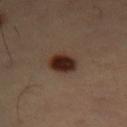Q: What is the anatomic site?
A: the left thigh
Q: Lesion size?
A: ≈3.5 mm
Q: How was this image acquired?
A: total-body-photography crop, ~15 mm field of view
Q: What lighting was used for the tile?
A: cross-polarized
Q: What did automated image analysis measure?
A: a footprint of about 7 mm², an outline eccentricity of about 0.6 (0 = round, 1 = elongated), and a symmetry-axis asymmetry near 0.15; an automated nevus-likeness rating near 100 out of 100
Q: Who is the patient?
A: male, approximately 50 years of age